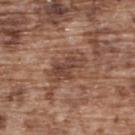Clinical impression: Part of a total-body skin-imaging series; this lesion was reviewed on a skin check and was not flagged for biopsy. Background: The subject is a male aged approximately 75. The tile uses white-light illumination. The recorded lesion diameter is about 4.5 mm. From the upper back. The lesion-visualizer software estimated a lesion–skin lightness drop of about 9 and a normalized lesion–skin contrast near 7.5. And it measured an automated nevus-likeness rating near 0 out of 100 and a detector confidence of about 80 out of 100 that the crop contains a lesion. A roughly 15 mm field-of-view crop from a total-body skin photograph.